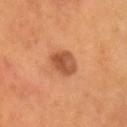Impression: This lesion was catalogued during total-body skin photography and was not selected for biopsy. Background: Automated image analysis of the tile measured a shape eccentricity near 0.7 and two-axis asymmetry of about 0.15. And it measured a mean CIELAB color near L≈47 a*≈25 b*≈35, about 11 CIELAB-L* units darker than the surrounding skin, and a normalized lesion–skin contrast near 8. And it measured internal color variation of about 4.5 on a 0–10 scale and peripheral color asymmetry of about 1.5. The recorded lesion diameter is about 3.5 mm. The patient is a male aged around 55. Captured under cross-polarized illumination. Located on the head or neck. Cropped from a whole-body photographic skin survey; the tile spans about 15 mm.